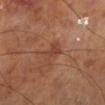Q: Is there a histopathology result?
A: no biopsy performed (imaged during a skin exam)
Q: What is the anatomic site?
A: the left lower leg
Q: How was this image acquired?
A: ~15 mm tile from a whole-body skin photo
Q: What are the patient's age and sex?
A: male, aged 68 to 72